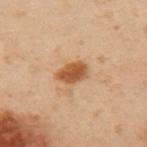A 15 mm close-up extracted from a 3D total-body photography capture.
The lesion is on the left upper arm.
The recorded lesion diameter is about 3 mm.
Captured under cross-polarized illumination.
The total-body-photography lesion software estimated lesion-presence confidence of about 100/100.
A male subject, aged approximately 55.